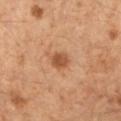The lesion was photographed on a routine skin check and not biopsied; there is no pathology result. Captured under cross-polarized illumination. Longest diameter approximately 2.5 mm. A close-up tile cropped from a whole-body skin photograph, about 15 mm across. A female patient about 55 years old. On the arm. The lesion-visualizer software estimated a footprint of about 4 mm², a shape eccentricity near 0.6, and a symmetry-axis asymmetry near 0.2. The software also gave an average lesion color of about L≈43 a*≈20 b*≈31 (CIELAB), about 10 CIELAB-L* units darker than the surrounding skin, and a normalized border contrast of about 8. The analysis additionally found a border-irregularity rating of about 1.5/10, internal color variation of about 2 on a 0–10 scale, and peripheral color asymmetry of about 1. The analysis additionally found a detector confidence of about 100 out of 100 that the crop contains a lesion.